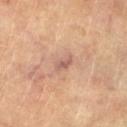biopsy status: no biopsy performed (imaged during a skin exam) | acquisition: ~15 mm tile from a whole-body skin photo | TBP lesion metrics: a lesion area of about 3 mm², an outline eccentricity of about 0.9 (0 = round, 1 = elongated), and two-axis asymmetry of about 0.3; a mean CIELAB color near L≈50 a*≈18 b*≈24, a lesion–skin lightness drop of about 8, and a normalized lesion–skin contrast near 6; a within-lesion color-variation index near 1/10 and peripheral color asymmetry of about 0 | diameter: ~3 mm (longest diameter) | patient: female, aged 78 to 82 | location: the left lower leg | lighting: cross-polarized.